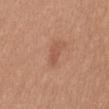The lesion was tiled from a total-body skin photograph and was not biopsied.
The tile uses white-light illumination.
A female patient aged approximately 40.
From the back.
Cropped from a whole-body photographic skin survey; the tile spans about 15 mm.
The lesion's longest dimension is about 2.5 mm.